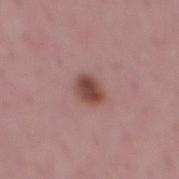No biopsy was performed on this lesion — it was imaged during a full skin examination and was not determined to be concerning. A 15 mm close-up tile from a total-body photography series done for melanoma screening. This is a white-light tile. From the mid back. Automated tile analysis of the lesion measured a lesion color around L≈46 a*≈23 b*≈23 in CIELAB, about 13 CIELAB-L* units darker than the surrounding skin, and a normalized lesion–skin contrast near 9.5. It also reported a border-irregularity rating of about 2/10 and a within-lesion color-variation index near 2.5/10. A male patient, approximately 50 years of age. The recorded lesion diameter is about 3 mm.